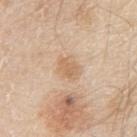Imaged during a routine full-body skin examination; the lesion was not biopsied and no histopathology is available. The tile uses white-light illumination. A region of skin cropped from a whole-body photographic capture, roughly 15 mm wide. A male subject aged approximately 80. Longest diameter approximately 3.5 mm. The lesion is located on the right upper arm.